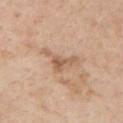lighting: white-light illumination | body site: the arm | image-analysis metrics: a footprint of about 6.5 mm² and a shape eccentricity near 0.8; a border-irregularity index near 8/10, a color-variation rating of about 4/10, and radial color variation of about 1.5 | patient: male, aged 58–62 | lesion size: ≈4.5 mm | imaging modality: ~15 mm crop, total-body skin-cancer survey.